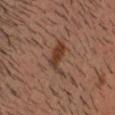{
  "biopsy_status": "not biopsied; imaged during a skin examination",
  "lighting": "cross-polarized",
  "lesion_size": {
    "long_diameter_mm_approx": 4.0
  },
  "image": {
    "source": "total-body photography crop",
    "field_of_view_mm": 15
  },
  "automated_metrics": {
    "area_mm2_approx": 6.0,
    "eccentricity": 0.85,
    "shape_asymmetry": 0.55,
    "border_irregularity_0_10": 6.5,
    "color_variation_0_10": 2.0,
    "peripheral_color_asymmetry": 1.0,
    "nevus_likeness_0_100": 95,
    "lesion_detection_confidence_0_100": 100
  },
  "patient": {
    "sex": "male",
    "age_approx": 40
  },
  "site": "head or neck"
}